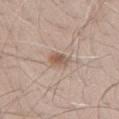Located on the right upper arm.
The recorded lesion diameter is about 2.5 mm.
Cropped from a whole-body photographic skin survey; the tile spans about 15 mm.
The total-body-photography lesion software estimated a normalized border contrast of about 7. It also reported a lesion-detection confidence of about 100/100.
The tile uses white-light illumination.
The patient is a male aged 68 to 72.